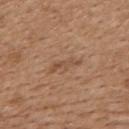{"biopsy_status": "not biopsied; imaged during a skin examination", "site": "upper back", "lesion_size": {"long_diameter_mm_approx": 4.0}, "patient": {"sex": "female", "age_approx": 45}, "lighting": "white-light", "automated_metrics": {"eccentricity": 0.95, "shape_asymmetry": 0.35, "vs_skin_darker_L": 7.0, "color_variation_0_10": 0.0, "peripheral_color_asymmetry": 0.0, "nevus_likeness_0_100": 0, "lesion_detection_confidence_0_100": 100}, "image": {"source": "total-body photography crop", "field_of_view_mm": 15}}